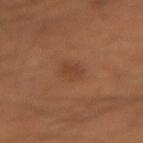biopsy status: imaged on a skin check; not biopsied
automated lesion analysis: an area of roughly 5.5 mm², an outline eccentricity of about 0.55 (0 = round, 1 = elongated), and a shape-asymmetry score of about 0.2 (0 = symmetric); an average lesion color of about L≈41 a*≈22 b*≈31 (CIELAB), roughly 6 lightness units darker than nearby skin, and a normalized border contrast of about 5; a border-irregularity rating of about 1.5/10 and a peripheral color-asymmetry measure near 0.5; an automated nevus-likeness rating near 65 out of 100 and a lesion-detection confidence of about 100/100
tile lighting: cross-polarized
patient: male, aged approximately 60
imaging modality: ~15 mm crop, total-body skin-cancer survey
diameter: ≈3 mm
site: the left forearm